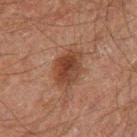notes = imaged on a skin check; not biopsied | patient = male, aged 58–62 | imaging modality = 15 mm crop, total-body photography | location = the right thigh.